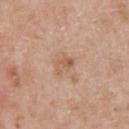{"biopsy_status": "not biopsied; imaged during a skin examination", "patient": {"sex": "male", "age_approx": 50}, "site": "chest", "lesion_size": {"long_diameter_mm_approx": 2.5}, "lighting": "white-light", "image": {"source": "total-body photography crop", "field_of_view_mm": 15}}